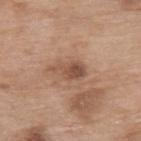Captured during whole-body skin photography for melanoma surveillance; the lesion was not biopsied.